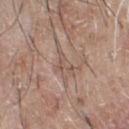{
  "biopsy_status": "not biopsied; imaged during a skin examination",
  "site": "chest",
  "patient": {
    "sex": "male",
    "age_approx": 65
  },
  "lighting": "white-light",
  "lesion_size": {
    "long_diameter_mm_approx": 2.5
  },
  "image": {
    "source": "total-body photography crop",
    "field_of_view_mm": 15
  }
}